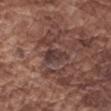Q: Is there a histopathology result?
A: imaged on a skin check; not biopsied
Q: Where on the body is the lesion?
A: the arm
Q: What kind of image is this?
A: ~15 mm crop, total-body skin-cancer survey
Q: What is the lesion's diameter?
A: about 3.5 mm
Q: Illumination type?
A: white-light
Q: Automated lesion metrics?
A: a lesion area of about 5 mm², an outline eccentricity of about 0.85 (0 = round, 1 = elongated), and a symmetry-axis asymmetry near 0.35; a lesion color around L≈36 a*≈18 b*≈19 in CIELAB, about 7 CIELAB-L* units darker than the surrounding skin, and a normalized lesion–skin contrast near 7.5; a border-irregularity rating of about 3.5/10, a within-lesion color-variation index near 3/10, and radial color variation of about 1
Q: Who is the patient?
A: male, roughly 75 years of age